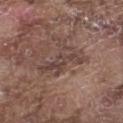automated lesion analysis: a shape eccentricity near 0.9 and a shape-asymmetry score of about 0.35 (0 = symmetric); internal color variation of about 5 on a 0–10 scale and a peripheral color-asymmetry measure near 2; a lesion-detection confidence of about 90/100 | diameter: ~5.5 mm (longest diameter) | body site: the left thigh | lighting: white-light illumination | imaging modality: 15 mm crop, total-body photography | subject: male, aged approximately 75.